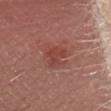This lesion was catalogued during total-body skin photography and was not selected for biopsy.